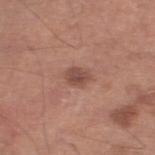lighting=white-light illumination
automated metrics=about 10 CIELAB-L* units darker than the surrounding skin; a border-irregularity index near 2.5/10, a within-lesion color-variation index near 2.5/10, and a peripheral color-asymmetry measure near 1; an automated nevus-likeness rating near 60 out of 100 and a detector confidence of about 100 out of 100 that the crop contains a lesion
imaging modality=~15 mm tile from a whole-body skin photo
patient=male, aged approximately 65
site=the leg